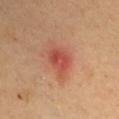Captured during whole-body skin photography for melanoma surveillance; the lesion was not biopsied.
A male patient about 55 years old.
The lesion's longest dimension is about 5 mm.
The lesion is on the left upper arm.
Cropped from a total-body skin-imaging series; the visible field is about 15 mm.